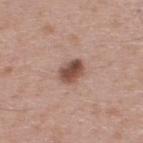biopsy status: total-body-photography surveillance lesion; no biopsy
patient: male, in their 40s
imaging modality: 15 mm crop, total-body photography
location: the upper back